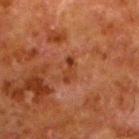| key | value |
|---|---|
| biopsy status | no biopsy performed (imaged during a skin exam) |
| size | about 3 mm |
| imaging modality | 15 mm crop, total-body photography |
| site | the left lower leg |
| patient | male, aged 78 to 82 |
| TBP lesion metrics | an area of roughly 3 mm², an eccentricity of roughly 0.95, and two-axis asymmetry of about 0.25; a lesion color around L≈31 a*≈23 b*≈29 in CIELAB, roughly 7 lightness units darker than nearby skin, and a lesion-to-skin contrast of about 7 (normalized; higher = more distinct); a border-irregularity index near 3.5/10 |
| illumination | cross-polarized illumination |